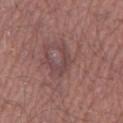notes = imaged on a skin check; not biopsied
tile lighting = white-light illumination
location = the left lower leg
patient = male, in their mid-50s
TBP lesion metrics = a classifier nevus-likeness of about 0/100 and a lesion-detection confidence of about 90/100
image source = 15 mm crop, total-body photography
size = ≈3.5 mm